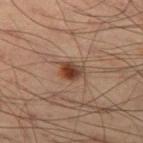Findings:
– follow-up: imaged on a skin check; not biopsied
– size: ~3 mm (longest diameter)
– patient: male, in their mid-50s
– image source: ~15 mm tile from a whole-body skin photo
– tile lighting: cross-polarized
– body site: the left thigh